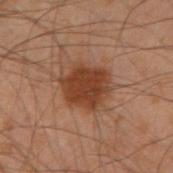A male subject, approximately 50 years of age. Captured under cross-polarized illumination. From the left arm. A roughly 15 mm field-of-view crop from a total-body skin photograph.